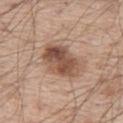Clinical impression: Part of a total-body skin-imaging series; this lesion was reviewed on a skin check and was not flagged for biopsy. Image and clinical context: The patient is a male about 60 years old. The lesion is on the left thigh. Cropped from a whole-body photographic skin survey; the tile spans about 15 mm.